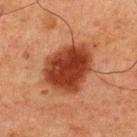{"biopsy_status": "not biopsied; imaged during a skin examination", "lighting": "cross-polarized", "image": {"source": "total-body photography crop", "field_of_view_mm": 15}, "lesion_size": {"long_diameter_mm_approx": 6.0}, "automated_metrics": {"area_mm2_approx": 24.0, "eccentricity": 0.6, "shape_asymmetry": 0.2, "color_variation_0_10": 4.0, "peripheral_color_asymmetry": 1.0, "lesion_detection_confidence_0_100": 100}, "site": "upper back", "patient": {"sex": "male", "age_approx": 60}}